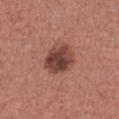Part of a total-body skin-imaging series; this lesion was reviewed on a skin check and was not flagged for biopsy. The subject is a female approximately 25 years of age. The recorded lesion diameter is about 4 mm. A region of skin cropped from a whole-body photographic capture, roughly 15 mm wide. Automated image analysis of the tile measured a lesion color around L≈42 a*≈23 b*≈24 in CIELAB, about 14 CIELAB-L* units darker than the surrounding skin, and a lesion-to-skin contrast of about 10 (normalized; higher = more distinct). The lesion is located on the chest.